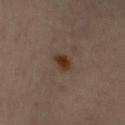{
  "biopsy_status": "not biopsied; imaged during a skin examination"
}